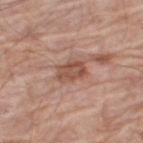Findings:
- workup — catalogued during a skin exam; not biopsied
- lesion size — ~3.5 mm (longest diameter)
- subject — male, about 80 years old
- tile lighting — white-light
- automated metrics — a lesion area of about 6.5 mm², a shape eccentricity near 0.8, and a shape-asymmetry score of about 0.25 (0 = symmetric); a border-irregularity rating of about 2.5/10, internal color variation of about 3.5 on a 0–10 scale, and a peripheral color-asymmetry measure near 1; a detector confidence of about 100 out of 100 that the crop contains a lesion
- imaging modality — 15 mm crop, total-body photography
- body site — the right thigh